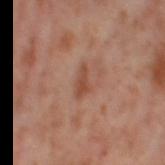No biopsy was performed on this lesion — it was imaged during a full skin examination and was not determined to be concerning.
This image is a 15 mm lesion crop taken from a total-body photograph.
A female patient, approximately 55 years of age.
On the left thigh.